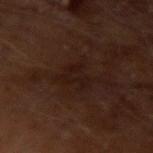- workup · no biopsy performed (imaged during a skin exam)
- illumination · cross-polarized illumination
- diameter · ≈3 mm
- anatomic site · the arm
- patient · male, in their mid- to late 60s
- acquisition · ~15 mm crop, total-body skin-cancer survey